A lesion tile, about 15 mm wide, cut from a 3D total-body photograph · the patient is a male about 70 years old · Automated tile analysis of the lesion measured an area of roughly 3.5 mm², an eccentricity of roughly 0.8, and two-axis asymmetry of about 0.25. The analysis additionally found a lesion color around L≈26 a*≈27 b*≈20 in CIELAB, roughly 5 lightness units darker than nearby skin, and a lesion-to-skin contrast of about 6 (normalized; higher = more distinct). The analysis additionally found a border-irregularity rating of about 2.5/10, a color-variation rating of about 1/10, and radial color variation of about 0.5 · about 2.5 mm across · captured under white-light illumination · the lesion is located on the arm.
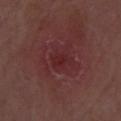Conclusion:
Histopathological examination showed a squamous cell carcinoma in situ, classified as a skin cancer.